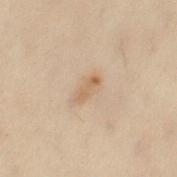{"biopsy_status": "not biopsied; imaged during a skin examination", "lighting": "cross-polarized", "lesion_size": {"long_diameter_mm_approx": 2.5}, "image": {"source": "total-body photography crop", "field_of_view_mm": 15}, "site": "left thigh", "patient": {"sex": "female", "age_approx": 50}}